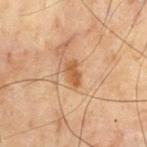The lesion was tiled from a total-body skin photograph and was not biopsied.
An algorithmic analysis of the crop reported a lesion area of about 4 mm² and an eccentricity of roughly 0.85. And it measured an automated nevus-likeness rating near 25 out of 100.
Cropped from a total-body skin-imaging series; the visible field is about 15 mm.
From the chest.
The lesion's longest dimension is about 3 mm.
Imaged with cross-polarized lighting.
A male subject, aged approximately 70.